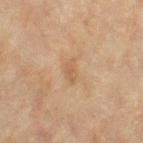Image and clinical context:
Measured at roughly 2.5 mm in maximum diameter. Cropped from a whole-body photographic skin survey; the tile spans about 15 mm. This is a cross-polarized tile. The patient is a female aged approximately 80. The lesion is located on the left thigh.